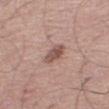location: the right thigh
image source: ~15 mm tile from a whole-body skin photo
patient: male, aged 68–72